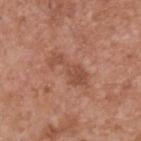Clinical impression: The lesion was photographed on a routine skin check and not biopsied; there is no pathology result. Background: Automated tile analysis of the lesion measured an area of roughly 8 mm², an outline eccentricity of about 0.9 (0 = round, 1 = elongated), and a shape-asymmetry score of about 0.45 (0 = symmetric). It also reported a lesion color around L≈50 a*≈24 b*≈30 in CIELAB, about 8 CIELAB-L* units darker than the surrounding skin, and a lesion-to-skin contrast of about 5.5 (normalized; higher = more distinct). A close-up tile cropped from a whole-body skin photograph, about 15 mm across. Longest diameter approximately 5 mm. The tile uses white-light illumination. The lesion is on the upper back. A male subject in their mid- to late 50s.